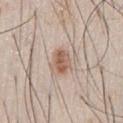Captured during whole-body skin photography for melanoma surveillance; the lesion was not biopsied.
The lesion is on the chest.
A 15 mm close-up tile from a total-body photography series done for melanoma screening.
A male patient, aged 43–47.